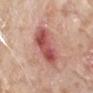Q: Was a biopsy performed?
A: total-body-photography surveillance lesion; no biopsy
Q: What did automated image analysis measure?
A: a lesion area of about 15 mm², an outline eccentricity of about 0.85 (0 = round, 1 = elongated), and a shape-asymmetry score of about 0.25 (0 = symmetric); a mean CIELAB color near L≈53 a*≈30 b*≈25, about 13 CIELAB-L* units darker than the surrounding skin, and a normalized lesion–skin contrast near 9; a border-irregularity rating of about 3/10, a color-variation rating of about 5.5/10, and radial color variation of about 2; a lesion-detection confidence of about 100/100
Q: Illumination type?
A: white-light
Q: Lesion size?
A: ~5.5 mm (longest diameter)
Q: What are the patient's age and sex?
A: male, aged around 75
Q: What kind of image is this?
A: ~15 mm tile from a whole-body skin photo
Q: What is the anatomic site?
A: the left upper arm